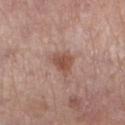biopsy status: imaged on a skin check; not biopsied | image source: ~15 mm crop, total-body skin-cancer survey | tile lighting: white-light illumination | diameter: about 3.5 mm | body site: the left lower leg | patient: female, approximately 50 years of age.